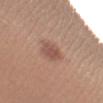| key | value |
|---|---|
| notes | catalogued during a skin exam; not biopsied |
| image | total-body-photography crop, ~15 mm field of view |
| patient | male, aged approximately 30 |
| site | the right lower leg |
| illumination | white-light illumination |
| lesion diameter | ~3 mm (longest diameter) |